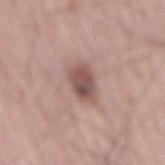The lesion was photographed on a routine skin check and not biopsied; there is no pathology result.
Longest diameter approximately 4 mm.
A roughly 15 mm field-of-view crop from a total-body skin photograph.
A male subject, aged 63 to 67.
The lesion is on the mid back.